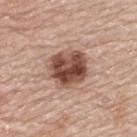The lesion was photographed on a routine skin check and not biopsied; there is no pathology result. Located on the upper back. A roughly 15 mm field-of-view crop from a total-body skin photograph. The patient is a male aged approximately 70.